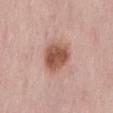notes: imaged on a skin check; not biopsied | lighting: white-light illumination | automated lesion analysis: an area of roughly 12 mm², an outline eccentricity of about 0.7 (0 = round, 1 = elongated), and a shape-asymmetry score of about 0.15 (0 = symmetric); a detector confidence of about 100 out of 100 that the crop contains a lesion | image: ~15 mm crop, total-body skin-cancer survey | subject: female, approximately 50 years of age | site: the back | lesion diameter: ≈4.5 mm.